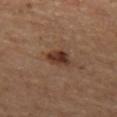lesion diameter: ≈3 mm | subject: male, aged 63–67 | lighting: cross-polarized illumination | body site: the left thigh | acquisition: ~15 mm tile from a whole-body skin photo | TBP lesion metrics: a footprint of about 5 mm², a shape eccentricity near 0.7, and a shape-asymmetry score of about 0.25 (0 = symmetric); a classifier nevus-likeness of about 85/100 and lesion-presence confidence of about 100/100.